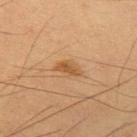<tbp_lesion>
  <biopsy_status>not biopsied; imaged during a skin examination</biopsy_status>
  <lighting>cross-polarized</lighting>
  <lesion_size>
    <long_diameter_mm_approx>3.0</long_diameter_mm_approx>
  </lesion_size>
  <image>
    <source>total-body photography crop</source>
    <field_of_view_mm>15</field_of_view_mm>
  </image>
  <patient>
    <sex>male</sex>
    <age_approx>35</age_approx>
  </patient>
  <automated_metrics>
    <border_irregularity_0_10>3.5</border_irregularity_0_10>
    <peripheral_color_asymmetry>0.5</peripheral_color_asymmetry>
    <lesion_detection_confidence_0_100>100</lesion_detection_confidence_0_100>
  </automated_metrics>
  <site>left upper arm</site>
</tbp_lesion>